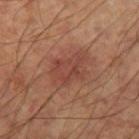Imaged during a routine full-body skin examination; the lesion was not biopsied and no histopathology is available.
A male subject about 60 years old.
Automated tile analysis of the lesion measured a lesion area of about 11 mm², an outline eccentricity of about 0.75 (0 = round, 1 = elongated), and a shape-asymmetry score of about 0.3 (0 = symmetric). It also reported a lesion color around L≈42 a*≈24 b*≈27 in CIELAB, roughly 7 lightness units darker than nearby skin, and a normalized lesion–skin contrast near 5.5. The analysis additionally found a border-irregularity rating of about 3.5/10 and a within-lesion color-variation index near 3/10.
The lesion is located on the right thigh.
A 15 mm close-up extracted from a 3D total-body photography capture.
The tile uses cross-polarized illumination.